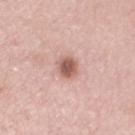follow-up = total-body-photography surveillance lesion; no biopsy